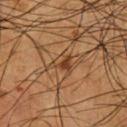{"biopsy_status": "not biopsied; imaged during a skin examination"}